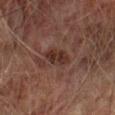Findings:
* workup — catalogued during a skin exam; not biopsied
* automated metrics — border irregularity of about 3 on a 0–10 scale and peripheral color asymmetry of about 1; a nevus-likeness score of about 30/100 and lesion-presence confidence of about 100/100
* site — the left lower leg
* acquisition — ~15 mm crop, total-body skin-cancer survey
* patient — male, aged approximately 75
* illumination — cross-polarized illumination
* lesion size — about 3.5 mm